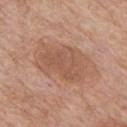| feature | finding |
|---|---|
| workup | no biopsy performed (imaged during a skin exam) |
| subject | male, aged 58 to 62 |
| tile lighting | white-light |
| acquisition | total-body-photography crop, ~15 mm field of view |
| site | the front of the torso |
| automated metrics | a footprint of about 19 mm², an eccentricity of roughly 0.75, and two-axis asymmetry of about 0.25 |
| lesion size | about 6 mm |